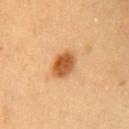Case summary:
• workup: no biopsy performed (imaged during a skin exam)
• lesion diameter: about 3.5 mm
• image source: ~15 mm crop, total-body skin-cancer survey
• anatomic site: the chest
• patient: female, about 30 years old